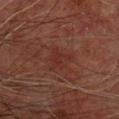The lesion was tiled from a total-body skin photograph and was not biopsied.
A lesion tile, about 15 mm wide, cut from a 3D total-body photograph.
A male patient roughly 60 years of age.
On the back.